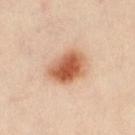Q: Is there a histopathology result?
A: no biopsy performed (imaged during a skin exam)
Q: What is the anatomic site?
A: the abdomen
Q: What kind of image is this?
A: ~15 mm tile from a whole-body skin photo
Q: Patient demographics?
A: female, roughly 40 years of age